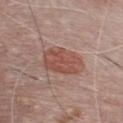biopsy_status: not biopsied; imaged during a skin examination
lighting: white-light
site: chest
image:
  source: total-body photography crop
  field_of_view_mm: 15
patient:
  sex: male
  age_approx: 75
lesion_size:
  long_diameter_mm_approx: 5.0
automated_metrics:
  border_irregularity_0_10: 2.5
  color_variation_0_10: 3.0
  peripheral_color_asymmetry: 1.0
  nevus_likeness_0_100: 95
  lesion_detection_confidence_0_100: 100